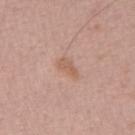This lesion was catalogued during total-body skin photography and was not selected for biopsy. Automated image analysis of the tile measured a lesion area of about 3 mm², an eccentricity of roughly 0.85, and two-axis asymmetry of about 0.3. The software also gave a border-irregularity index near 3.5/10, a color-variation rating of about 1/10, and a peripheral color-asymmetry measure near 0. The software also gave a nevus-likeness score of about 25/100 and a detector confidence of about 100 out of 100 that the crop contains a lesion. Cropped from a total-body skin-imaging series; the visible field is about 15 mm. A male patient, aged around 40. Measured at roughly 2.5 mm in maximum diameter. The lesion is located on the left forearm. The tile uses white-light illumination.